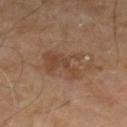An algorithmic analysis of the crop reported a footprint of about 12 mm², an outline eccentricity of about 0.65 (0 = round, 1 = elongated), and a symmetry-axis asymmetry near 0.25. The analysis additionally found a border-irregularity rating of about 4/10, a within-lesion color-variation index near 4.5/10, and radial color variation of about 1.5. And it measured an automated nevus-likeness rating near 0 out of 100 and lesion-presence confidence of about 100/100.
Cropped from a total-body skin-imaging series; the visible field is about 15 mm.
A male subject aged approximately 55.
This is a cross-polarized tile.
Measured at roughly 4.5 mm in maximum diameter.
On the right lower leg.